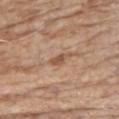{"biopsy_status": "not biopsied; imaged during a skin examination", "image": {"source": "total-body photography crop", "field_of_view_mm": 15}, "patient": {"sex": "male", "age_approx": "85+"}, "lighting": "white-light", "lesion_size": {"long_diameter_mm_approx": 2.5}, "automated_metrics": {"border_irregularity_0_10": 3.0, "nevus_likeness_0_100": 20}, "site": "chest"}